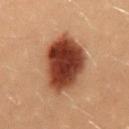follow-up = total-body-photography surveillance lesion; no biopsy
lighting = cross-polarized illumination
image-analysis metrics = an average lesion color of about L≈31 a*≈21 b*≈26 (CIELAB) and a normalized border contrast of about 14.5; a border-irregularity rating of about 2/10, internal color variation of about 6 on a 0–10 scale, and peripheral color asymmetry of about 2; a classifier nevus-likeness of about 100/100
diameter = about 7 mm
body site = the mid back
image source = ~15 mm crop, total-body skin-cancer survey
subject = female, aged approximately 20